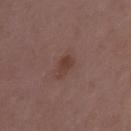follow-up: imaged on a skin check; not biopsied | tile lighting: white-light illumination | automated lesion analysis: a mean CIELAB color near L≈39 a*≈20 b*≈23, about 7 CIELAB-L* units darker than the surrounding skin, and a normalized lesion–skin contrast near 7; a border-irregularity index near 2/10 and a within-lesion color-variation index near 2.5/10; an automated nevus-likeness rating near 85 out of 100 | size: ~2.5 mm (longest diameter) | image source: ~15 mm tile from a whole-body skin photo | location: the left upper arm | subject: female, approximately 30 years of age.